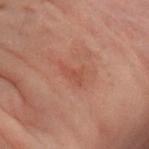| feature | finding |
|---|---|
| notes | imaged on a skin check; not biopsied |
| location | the right arm |
| diameter | ≈3 mm |
| subject | female, in their 60s |
| imaging modality | total-body-photography crop, ~15 mm field of view |
| illumination | cross-polarized |